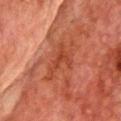workup: no biopsy performed (imaged during a skin exam) | anatomic site: the chest | subject: male, roughly 75 years of age | imaging modality: ~15 mm crop, total-body skin-cancer survey | diameter: ≈5 mm | automated metrics: a footprint of about 7 mm², an outline eccentricity of about 0.9 (0 = round, 1 = elongated), and a shape-asymmetry score of about 0.65 (0 = symmetric); a mean CIELAB color near L≈37 a*≈28 b*≈31, a lesion–skin lightness drop of about 7, and a normalized lesion–skin contrast near 6.5; border irregularity of about 8.5 on a 0–10 scale, a within-lesion color-variation index near 1.5/10, and peripheral color asymmetry of about 0.5 | tile lighting: cross-polarized illumination.